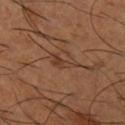Q: What is the anatomic site?
A: the right lower leg
Q: Patient demographics?
A: male, roughly 65 years of age
Q: What kind of image is this?
A: 15 mm crop, total-body photography